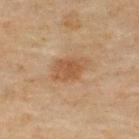Q: Is there a histopathology result?
A: no biopsy performed (imaged during a skin exam)
Q: What is the imaging modality?
A: 15 mm crop, total-body photography
Q: What are the patient's age and sex?
A: male, in their mid-40s
Q: What is the lesion's diameter?
A: ≈4.5 mm
Q: What is the anatomic site?
A: the upper back